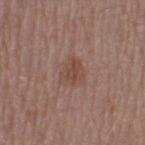<tbp_lesion>
  <biopsy_status>not biopsied; imaged during a skin examination</biopsy_status>
  <patient>
    <sex>female</sex>
    <age_approx>55</age_approx>
  </patient>
  <site>leg</site>
  <image>
    <source>total-body photography crop</source>
    <field_of_view_mm>15</field_of_view_mm>
  </image>
</tbp_lesion>